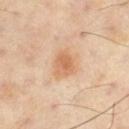Captured during whole-body skin photography for melanoma surveillance; the lesion was not biopsied. A 15 mm close-up extracted from a 3D total-body photography capture. A male subject, in their mid- to late 50s. Located on the right thigh.